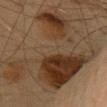Assessment:
Recorded during total-body skin imaging; not selected for excision or biopsy.
Context:
Automated image analysis of the tile measured a lesion area of about 75 mm², an outline eccentricity of about 0.6 (0 = round, 1 = elongated), and two-axis asymmetry of about 0.6. It also reported a mean CIELAB color near L≈33 a*≈14 b*≈26, a lesion–skin lightness drop of about 9, and a lesion-to-skin contrast of about 8.5 (normalized; higher = more distinct). On the back. A male subject, approximately 60 years of age. Measured at roughly 13 mm in maximum diameter. A region of skin cropped from a whole-body photographic capture, roughly 15 mm wide. Imaged with cross-polarized lighting.